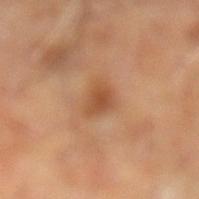{
  "biopsy_status": "not biopsied; imaged during a skin examination",
  "lighting": "cross-polarized",
  "automated_metrics": {
    "nevus_likeness_0_100": 5,
    "lesion_detection_confidence_0_100": 100
  },
  "lesion_size": {
    "long_diameter_mm_approx": 3.0
  },
  "image": {
    "source": "total-body photography crop",
    "field_of_view_mm": 15
  },
  "site": "left lower leg",
  "patient": {
    "sex": "male",
    "age_approx": 70
  }
}